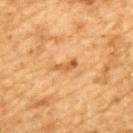Assessment: The lesion was photographed on a routine skin check and not biopsied; there is no pathology result. Background: A region of skin cropped from a whole-body photographic capture, roughly 15 mm wide. A male subject aged around 85 or older. The lesion is on the back. The tile uses cross-polarized illumination. Longest diameter approximately 3 mm.